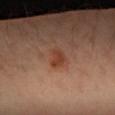Case summary:
• follow-up · imaged on a skin check; not biopsied
• acquisition · total-body-photography crop, ~15 mm field of view
• automated metrics · a lesion area of about 4 mm² and a shape eccentricity near 0.85; border irregularity of about 2.5 on a 0–10 scale; a lesion-detection confidence of about 100/100
• subject · female, aged around 40
• lesion diameter · ≈3 mm
• location · the arm
• tile lighting · cross-polarized